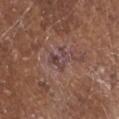The lesion was tiled from a total-body skin photograph and was not biopsied.
A male subject aged 78 to 82.
The lesion-visualizer software estimated border irregularity of about 6 on a 0–10 scale, a color-variation rating of about 3.5/10, and a peripheral color-asymmetry measure near 1. It also reported lesion-presence confidence of about 80/100.
Longest diameter approximately 2.5 mm.
The tile uses white-light illumination.
Cropped from a total-body skin-imaging series; the visible field is about 15 mm.
Located on the head or neck.